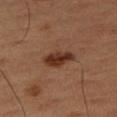<case>
  <biopsy_status>not biopsied; imaged during a skin examination</biopsy_status>
  <site>leg</site>
  <image>
    <source>total-body photography crop</source>
    <field_of_view_mm>15</field_of_view_mm>
  </image>
  <patient>
    <sex>male</sex>
    <age_approx>50</age_approx>
  </patient>
</case>